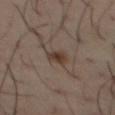biopsy status — no biopsy performed (imaged during a skin exam)
size — ~2.5 mm (longest diameter)
body site — the chest
image source — ~15 mm crop, total-body skin-cancer survey
patient — male, aged around 40
TBP lesion metrics — a footprint of about 4 mm², an eccentricity of roughly 0.65, and a symmetry-axis asymmetry near 0.25; an average lesion color of about L≈36 a*≈13 b*≈22 (CIELAB) and roughly 9 lightness units darker than nearby skin; a border-irregularity index near 2.5/10, internal color variation of about 3.5 on a 0–10 scale, and a peripheral color-asymmetry measure near 1; a detector confidence of about 100 out of 100 that the crop contains a lesion